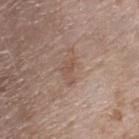Clinical impression:
The lesion was photographed on a routine skin check and not biopsied; there is no pathology result.
Clinical summary:
Approximately 3 mm at its widest. A female subject, about 75 years old. Cropped from a total-body skin-imaging series; the visible field is about 15 mm. Imaged with white-light lighting. From the upper back. An algorithmic analysis of the crop reported a border-irregularity rating of about 5.5/10 and internal color variation of about 0 on a 0–10 scale. It also reported an automated nevus-likeness rating near 0 out of 100.